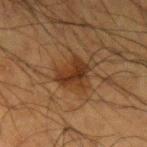Clinical impression: Part of a total-body skin-imaging series; this lesion was reviewed on a skin check and was not flagged for biopsy. Image and clinical context: A lesion tile, about 15 mm wide, cut from a 3D total-body photograph. The subject is a male aged approximately 60. The tile uses cross-polarized illumination. The lesion is on the left upper arm. Measured at roughly 3.5 mm in maximum diameter.